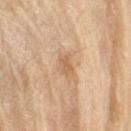automated_metrics:
  cielab_L: 62
  cielab_a: 19
  cielab_b: 36
  vs_skin_darker_L: 9.0
  vs_skin_contrast_norm: 6.0
  border_irregularity_0_10: 2.5
  color_variation_0_10: 1.0
  peripheral_color_asymmetry: 0.5
patient:
  sex: male
  age_approx: 70
site: right upper arm
lighting: white-light
image:
  source: total-body photography crop
  field_of_view_mm: 15